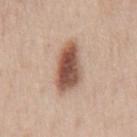Case summary:
• biopsy status: total-body-photography surveillance lesion; no biopsy
• location: the front of the torso
• size: about 6 mm
• subject: male, roughly 70 years of age
• tile lighting: white-light
• image: ~15 mm crop, total-body skin-cancer survey
• automated lesion analysis: a lesion area of about 15 mm² and an outline eccentricity of about 0.85 (0 = round, 1 = elongated); a color-variation rating of about 6.5/10 and peripheral color asymmetry of about 2